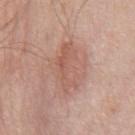notes: total-body-photography surveillance lesion; no biopsy | automated metrics: a mean CIELAB color near L≈57 a*≈22 b*≈27, about 8 CIELAB-L* units darker than the surrounding skin, and a lesion-to-skin contrast of about 5.5 (normalized; higher = more distinct); a color-variation rating of about 3.5/10 | lesion size: ~5.5 mm (longest diameter) | location: the chest | acquisition: ~15 mm crop, total-body skin-cancer survey | lighting: white-light | subject: male, aged approximately 70.